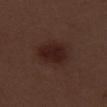{
  "biopsy_status": "not biopsied; imaged during a skin examination",
  "patient": {
    "sex": "male",
    "age_approx": 70
  },
  "automated_metrics": {
    "nevus_likeness_0_100": 95
  },
  "site": "right thigh",
  "image": {
    "source": "total-body photography crop",
    "field_of_view_mm": 15
  },
  "lighting": "white-light"
}